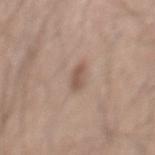biopsy status: no biopsy performed (imaged during a skin exam)
lighting: white-light
site: the mid back
patient: male, in their 60s
lesion diameter: ≈2.5 mm
imaging modality: ~15 mm crop, total-body skin-cancer survey
TBP lesion metrics: a footprint of about 3 mm², a shape eccentricity near 0.9, and two-axis asymmetry of about 0.3; a color-variation rating of about 0/10 and peripheral color asymmetry of about 0; a classifier nevus-likeness of about 35/100